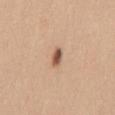Findings:
– notes — no biopsy performed (imaged during a skin exam)
– location — the mid back
– lighting — white-light illumination
– image source — ~15 mm crop, total-body skin-cancer survey
– subject — female, aged 28 to 32
– lesion diameter — about 2.5 mm